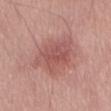Impression: This lesion was catalogued during total-body skin photography and was not selected for biopsy. Context: Automated tile analysis of the lesion measured a lesion-to-skin contrast of about 6 (normalized; higher = more distinct). It also reported a border-irregularity index near 3.5/10 and a peripheral color-asymmetry measure near 1. It also reported lesion-presence confidence of about 100/100. Imaged with white-light lighting. On the abdomen. A close-up tile cropped from a whole-body skin photograph, about 15 mm across. A male patient, roughly 60 years of age. The recorded lesion diameter is about 6.5 mm.